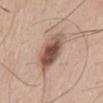Q: Was this lesion biopsied?
A: no biopsy performed (imaged during a skin exam)
Q: What is the lesion's diameter?
A: about 5.5 mm
Q: Patient demographics?
A: male, aged approximately 50
Q: How was the tile lit?
A: white-light
Q: Lesion location?
A: the chest
Q: How was this image acquired?
A: 15 mm crop, total-body photography
Q: What did automated image analysis measure?
A: a footprint of about 13 mm², an outline eccentricity of about 0.85 (0 = round, 1 = elongated), and a symmetry-axis asymmetry near 0.15; border irregularity of about 2 on a 0–10 scale, a within-lesion color-variation index near 8/10, and radial color variation of about 2.5